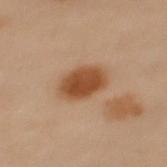Q: Was a biopsy performed?
A: no biopsy performed (imaged during a skin exam)